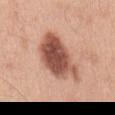Q: Was this lesion biopsied?
A: no biopsy performed (imaged during a skin exam)
Q: What is the anatomic site?
A: the mid back
Q: How was this image acquired?
A: 15 mm crop, total-body photography
Q: Automated lesion metrics?
A: a shape-asymmetry score of about 0.3 (0 = symmetric); an average lesion color of about L≈53 a*≈25 b*≈29 (CIELAB), about 17 CIELAB-L* units darker than the surrounding skin, and a normalized border contrast of about 11
Q: What are the patient's age and sex?
A: male, aged 43 to 47
Q: Illumination type?
A: white-light